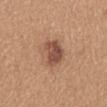Imaged during a routine full-body skin examination; the lesion was not biopsied and no histopathology is available.